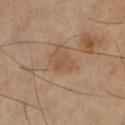Imaged during a routine full-body skin examination; the lesion was not biopsied and no histopathology is available.
A 15 mm close-up tile from a total-body photography series done for melanoma screening.
From the left lower leg.
The subject is a female roughly 70 years of age.
The tile uses cross-polarized illumination.
About 3 mm across.